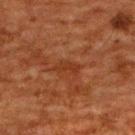Imaged during a routine full-body skin examination; the lesion was not biopsied and no histopathology is available. Captured under cross-polarized illumination. The recorded lesion diameter is about 3 mm. Located on the upper back. A male subject, about 60 years old. Cropped from a whole-body photographic skin survey; the tile spans about 15 mm.